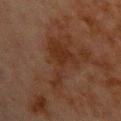This lesion was catalogued during total-body skin photography and was not selected for biopsy. The total-body-photography lesion software estimated a lesion area of about 13 mm², an eccentricity of roughly 0.85, and a shape-asymmetry score of about 0.7 (0 = symmetric). About 6.5 mm across. The subject is a male in their 70s. On the chest. A close-up tile cropped from a whole-body skin photograph, about 15 mm across.